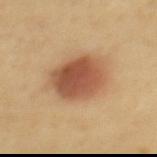Q: What is the anatomic site?
A: the upper back
Q: Illumination type?
A: cross-polarized
Q: Automated lesion metrics?
A: a lesion area of about 19 mm², an outline eccentricity of about 0.75 (0 = round, 1 = elongated), and a shape-asymmetry score of about 0.1 (0 = symmetric); an average lesion color of about L≈54 a*≈23 b*≈34 (CIELAB) and a lesion–skin lightness drop of about 14; a within-lesion color-variation index near 5.5/10 and peripheral color asymmetry of about 1.5; a nevus-likeness score of about 100/100
Q: How was this image acquired?
A: 15 mm crop, total-body photography
Q: What are the patient's age and sex?
A: female, aged approximately 60
Q: What is the lesion's diameter?
A: about 6 mm